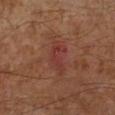follow-up — imaged on a skin check; not biopsied
diameter — about 4 mm
location — the left lower leg
automated metrics — a mean CIELAB color near L≈33 a*≈24 b*≈23, about 5 CIELAB-L* units darker than the surrounding skin, and a lesion-to-skin contrast of about 5.5 (normalized; higher = more distinct); a border-irregularity index near 5.5/10 and a within-lesion color-variation index near 2.5/10
tile lighting — cross-polarized illumination
patient — male, aged approximately 60
image source — 15 mm crop, total-body photography